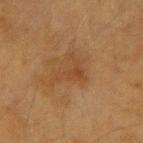• automated metrics — an area of roughly 6 mm² and a shape eccentricity near 0.7; a mean CIELAB color near L≈36 a*≈17 b*≈30, roughly 5 lightness units darker than nearby skin, and a normalized border contrast of about 5
• imaging modality — 15 mm crop, total-body photography
• illumination — cross-polarized
• subject — female, aged around 55
• size — ≈4 mm
• site — the left forearm